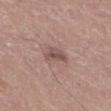Notes:
– illumination · white-light illumination
– lesion diameter · about 3.5 mm
– image-analysis metrics · a lesion area of about 4.5 mm², a shape eccentricity near 0.85, and two-axis asymmetry of about 0.35; an average lesion color of about L≈51 a*≈19 b*≈22 (CIELAB) and a normalized border contrast of about 7; a border-irregularity index near 4/10 and a peripheral color-asymmetry measure near 1
– anatomic site · the left lower leg
– imaging modality · total-body-photography crop, ~15 mm field of view
– patient · male, aged 43–47